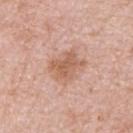<tbp_lesion>
<biopsy_status>not biopsied; imaged during a skin examination</biopsy_status>
<image>
  <source>total-body photography crop</source>
  <field_of_view_mm>15</field_of_view_mm>
</image>
<lesion_size>
  <long_diameter_mm_approx>4.0</long_diameter_mm_approx>
</lesion_size>
<site>upper back</site>
<lighting>white-light</lighting>
<patient>
  <sex>female</sex>
  <age_approx>45</age_approx>
</patient>
</tbp_lesion>